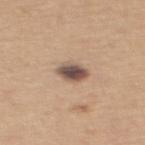Case summary:
• biopsy status · no biopsy performed (imaged during a skin exam)